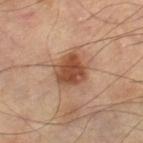Imaged during a routine full-body skin examination; the lesion was not biopsied and no histopathology is available. On the right thigh. Captured under cross-polarized illumination. A region of skin cropped from a whole-body photographic capture, roughly 15 mm wide. Automated tile analysis of the lesion measured a lesion color around L≈51 a*≈23 b*≈32 in CIELAB, about 13 CIELAB-L* units darker than the surrounding skin, and a normalized border contrast of about 9.5. The software also gave a nevus-likeness score of about 95/100 and lesion-presence confidence of about 100/100.